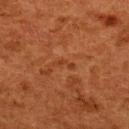Imaged during a routine full-body skin examination; the lesion was not biopsied and no histopathology is available.
A roughly 15 mm field-of-view crop from a total-body skin photograph.
The lesion is on the upper back.
A female subject roughly 50 years of age.